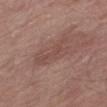Case summary:
• follow-up: no biopsy performed (imaged during a skin exam)
• patient: male, about 65 years old
• location: the leg
• image source: total-body-photography crop, ~15 mm field of view